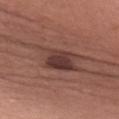biopsy status: total-body-photography surveillance lesion; no biopsy
automated metrics: a lesion–skin lightness drop of about 10 and a lesion-to-skin contrast of about 9 (normalized; higher = more distinct); a color-variation rating of about 5.5/10
anatomic site: the left upper arm
lighting: white-light illumination
lesion size: about 7 mm
patient: female, approximately 50 years of age
image: ~15 mm crop, total-body skin-cancer survey